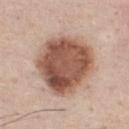| feature | finding |
|---|---|
| follow-up | no biopsy performed (imaged during a skin exam) |
| patient | male, about 45 years old |
| illumination | white-light illumination |
| acquisition | total-body-photography crop, ~15 mm field of view |
| anatomic site | the chest |
| automated lesion analysis | a lesion area of about 37 mm² and an outline eccentricity of about 0.4 (0 = round, 1 = elongated); internal color variation of about 6.5 on a 0–10 scale and peripheral color asymmetry of about 2; an automated nevus-likeness rating near 60 out of 100 and lesion-presence confidence of about 100/100 |
| lesion size | ~7 mm (longest diameter) |